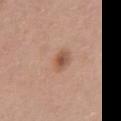follow-up: catalogued during a skin exam; not biopsied | image source: 15 mm crop, total-body photography | lesion size: ~4 mm (longest diameter) | automated lesion analysis: a footprint of about 9.5 mm², an outline eccentricity of about 0.7 (0 = round, 1 = elongated), and a shape-asymmetry score of about 0.2 (0 = symmetric); a border-irregularity rating of about 2/10 and a within-lesion color-variation index near 6/10; a classifier nevus-likeness of about 85/100 and a lesion-detection confidence of about 100/100 | body site: the front of the torso | subject: female, aged 58–62.